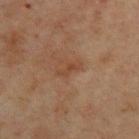No biopsy was performed on this lesion — it was imaged during a full skin examination and was not determined to be concerning.
A region of skin cropped from a whole-body photographic capture, roughly 15 mm wide.
A male patient roughly 45 years of age.
On the back.